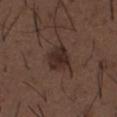notes: catalogued during a skin exam; not biopsied | illumination: white-light | image: 15 mm crop, total-body photography | diameter: about 3.5 mm | subject: male, roughly 50 years of age | body site: the abdomen.